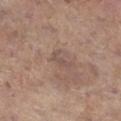Q: Is there a histopathology result?
A: total-body-photography surveillance lesion; no biopsy
Q: What are the patient's age and sex?
A: female, aged 83 or older
Q: What kind of image is this?
A: total-body-photography crop, ~15 mm field of view
Q: What is the anatomic site?
A: the right lower leg
Q: What lighting was used for the tile?
A: white-light illumination
Q: What is the lesion's diameter?
A: ~3 mm (longest diameter)